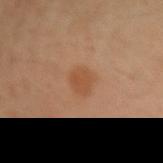Q: Is there a histopathology result?
A: no biopsy performed (imaged during a skin exam)
Q: What kind of image is this?
A: 15 mm crop, total-body photography
Q: Lesion size?
A: ≈2.5 mm
Q: Where on the body is the lesion?
A: the arm
Q: Who is the patient?
A: female, roughly 40 years of age